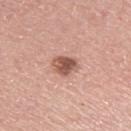Captured during whole-body skin photography for melanoma surveillance; the lesion was not biopsied.
Longest diameter approximately 3.5 mm.
This image is a 15 mm lesion crop taken from a total-body photograph.
The lesion is on the arm.
The total-body-photography lesion software estimated a mean CIELAB color near L≈55 a*≈23 b*≈26, about 14 CIELAB-L* units darker than the surrounding skin, and a normalized border contrast of about 9.5.
The subject is a female aged approximately 55.
The tile uses white-light illumination.